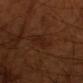follow-up — total-body-photography surveillance lesion; no biopsy
acquisition — ~15 mm tile from a whole-body skin photo
lesion diameter — ≈3 mm
lighting — cross-polarized
patient — male, roughly 70 years of age
location — the right upper arm